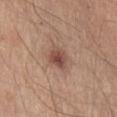Approximately 2.5 mm at its widest. The tile uses white-light illumination. Automated tile analysis of the lesion measured a footprint of about 5.5 mm². The analysis additionally found a border-irregularity index near 2/10, internal color variation of about 4.5 on a 0–10 scale, and a peripheral color-asymmetry measure near 1.5. And it measured an automated nevus-likeness rating near 80 out of 100 and lesion-presence confidence of about 100/100. The lesion is located on the front of the torso. A female patient, roughly 55 years of age. A 15 mm crop from a total-body photograph taken for skin-cancer surveillance.